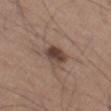Q: Was this lesion biopsied?
A: total-body-photography surveillance lesion; no biopsy
Q: What lighting was used for the tile?
A: white-light illumination
Q: What did automated image analysis measure?
A: internal color variation of about 5.5 on a 0–10 scale and radial color variation of about 2
Q: How was this image acquired?
A: total-body-photography crop, ~15 mm field of view
Q: What are the patient's age and sex?
A: male, approximately 65 years of age
Q: How large is the lesion?
A: ≈3.5 mm
Q: Where on the body is the lesion?
A: the leg